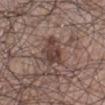workup = no biopsy performed (imaged during a skin exam); tile lighting = white-light illumination; anatomic site = the left lower leg; subject = male, aged approximately 60; lesion diameter = about 4 mm; acquisition = total-body-photography crop, ~15 mm field of view.